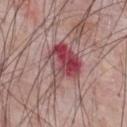Imaged during a routine full-body skin examination; the lesion was not biopsied and no histopathology is available.